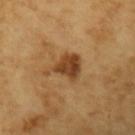The lesion was tiled from a total-body skin photograph and was not biopsied.
From the left upper arm.
A female subject roughly 70 years of age.
A close-up tile cropped from a whole-body skin photograph, about 15 mm across.